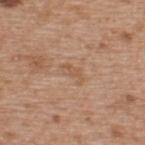Impression: Imaged during a routine full-body skin examination; the lesion was not biopsied and no histopathology is available. Background: A male patient in their mid-60s. Imaged with white-light lighting. A region of skin cropped from a whole-body photographic capture, roughly 15 mm wide. The recorded lesion diameter is about 3 mm. The lesion is on the upper back.